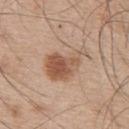Clinical summary:
The lesion is on the upper back. A male subject, roughly 55 years of age. A 15 mm close-up extracted from a 3D total-body photography capture.